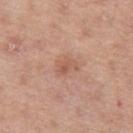biopsy status=catalogued during a skin exam; not biopsied
acquisition=15 mm crop, total-body photography
site=the leg
patient=female, aged 43 to 47